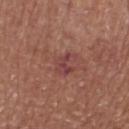Part of a total-body skin-imaging series; this lesion was reviewed on a skin check and was not flagged for biopsy. The lesion is on the leg. A male patient aged 73 to 77. This image is a 15 mm lesion crop taken from a total-body photograph. This is a white-light tile.